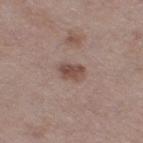Impression:
Part of a total-body skin-imaging series; this lesion was reviewed on a skin check and was not flagged for biopsy.
Clinical summary:
A female patient, approximately 50 years of age. A close-up tile cropped from a whole-body skin photograph, about 15 mm across. Longest diameter approximately 3 mm. The lesion is located on the right thigh. The tile uses white-light illumination.